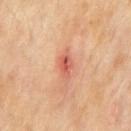| feature | finding |
|---|---|
| workup | catalogued during a skin exam; not biopsied |
| imaging modality | 15 mm crop, total-body photography |
| subject | male, aged around 70 |
| diameter | ~2.5 mm (longest diameter) |
| anatomic site | the chest |
| lighting | cross-polarized illumination |